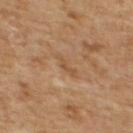Notes:
* workup · imaged on a skin check; not biopsied
* site · the upper back
* image · ~15 mm crop, total-body skin-cancer survey
* patient · female, aged 58 to 62
* illumination · cross-polarized illumination
* automated metrics · a lesion area of about 3 mm², a shape eccentricity near 0.9, and two-axis asymmetry of about 0.35; a mean CIELAB color near L≈47 a*≈18 b*≈32, a lesion–skin lightness drop of about 6, and a normalized lesion–skin contrast near 5
* diameter · ~3 mm (longest diameter)